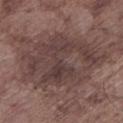Case summary:
* notes: no biopsy performed (imaged during a skin exam)
* subject: male, roughly 75 years of age
* body site: the left lower leg
* imaging modality: 15 mm crop, total-body photography
* diameter: ≈11 mm
* lighting: white-light
* automated lesion analysis: roughly 8 lightness units darker than nearby skin; a border-irregularity index near 3.5/10, internal color variation of about 5.5 on a 0–10 scale, and peripheral color asymmetry of about 2